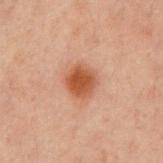The lesion was photographed on a routine skin check and not biopsied; there is no pathology result.
This is a cross-polarized tile.
The lesion's longest dimension is about 3 mm.
A region of skin cropped from a whole-body photographic capture, roughly 15 mm wide.
From the chest.
The patient is a male about 60 years old.
The total-body-photography lesion software estimated a border-irregularity rating of about 1.5/10 and a within-lesion color-variation index near 3/10. The analysis additionally found a nevus-likeness score of about 100/100 and lesion-presence confidence of about 100/100.